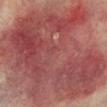follow-up: no biopsy performed (imaged during a skin exam) | acquisition: 15 mm crop, total-body photography | body site: the left lower leg | diameter: ~17.5 mm (longest diameter) | subject: male, aged approximately 75 | automated metrics: an area of roughly 175 mm², a shape eccentricity near 0.7, and two-axis asymmetry of about 0.15; a mean CIELAB color near L≈40 a*≈24 b*≈20, a lesion–skin lightness drop of about 11, and a lesion-to-skin contrast of about 9 (normalized; higher = more distinct); a border-irregularity rating of about 2.5/10, internal color variation of about 7.5 on a 0–10 scale, and peripheral color asymmetry of about 2.5.